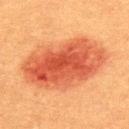| feature | finding |
|---|---|
| biopsy status | catalogued during a skin exam; not biopsied |
| TBP lesion metrics | a lesion area of about 41 mm², an outline eccentricity of about 0.85 (0 = round, 1 = elongated), and a shape-asymmetry score of about 0.15 (0 = symmetric); internal color variation of about 6 on a 0–10 scale; a nevus-likeness score of about 100/100 and lesion-presence confidence of about 100/100 |
| image | 15 mm crop, total-body photography |
| location | the upper back |
| tile lighting | cross-polarized |
| diameter | ~10 mm (longest diameter) |
| subject | male, aged approximately 40 |